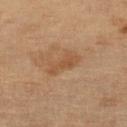Case summary:
* workup: no biopsy performed (imaged during a skin exam)
* image-analysis metrics: a border-irregularity rating of about 5/10
* diameter: about 4 mm
* lighting: cross-polarized illumination
* imaging modality: 15 mm crop, total-body photography
* subject: female, roughly 55 years of age
* anatomic site: the left lower leg